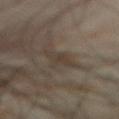Q: Was a biopsy performed?
A: imaged on a skin check; not biopsied
Q: How large is the lesion?
A: about 3.5 mm
Q: What did automated image analysis measure?
A: an eccentricity of roughly 0.8 and a symmetry-axis asymmetry near 0.45; a lesion color around L≈36 a*≈9 b*≈21 in CIELAB, roughly 6 lightness units darker than nearby skin, and a normalized lesion–skin contrast near 6; a border-irregularity index near 5/10, a within-lesion color-variation index near 2/10, and radial color variation of about 0.5; a lesion-detection confidence of about 70/100
Q: What kind of image is this?
A: total-body-photography crop, ~15 mm field of view
Q: Illumination type?
A: cross-polarized illumination
Q: Where on the body is the lesion?
A: the abdomen
Q: What are the patient's age and sex?
A: male, roughly 60 years of age